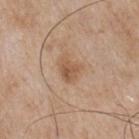Imaged during a routine full-body skin examination; the lesion was not biopsied and no histopathology is available. A 15 mm close-up tile from a total-body photography series done for melanoma screening. Automated image analysis of the tile measured a mean CIELAB color near L≈55 a*≈19 b*≈33, about 9 CIELAB-L* units darker than the surrounding skin, and a normalized lesion–skin contrast near 6.5. And it measured a border-irregularity rating of about 3.5/10, a within-lesion color-variation index near 4/10, and radial color variation of about 1.5. The tile uses white-light illumination. A male subject, aged 63 to 67. From the chest.